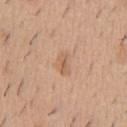Notes:
* workup — no biopsy performed (imaged during a skin exam)
* body site — the front of the torso
* subject — male, aged 38 to 42
* acquisition — 15 mm crop, total-body photography
* lighting — white-light
* lesion diameter — about 2.5 mm